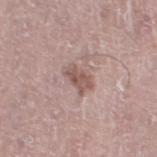Recorded during total-body skin imaging; not selected for excision or biopsy. The lesion is on the left thigh. A male subject in their mid- to late 70s. Cropped from a whole-body photographic skin survey; the tile spans about 15 mm. Imaged with white-light lighting.